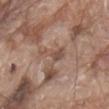Assessment:
No biopsy was performed on this lesion — it was imaged during a full skin examination and was not determined to be concerning.
Clinical summary:
A male patient, about 80 years old. Located on the abdomen. Cropped from a total-body skin-imaging series; the visible field is about 15 mm. Measured at roughly 3.5 mm in maximum diameter.